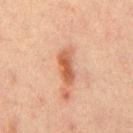Clinical impression: Part of a total-body skin-imaging series; this lesion was reviewed on a skin check and was not flagged for biopsy. Image and clinical context: A 15 mm close-up extracted from a 3D total-body photography capture. Measured at roughly 4.5 mm in maximum diameter. The total-body-photography lesion software estimated a lesion area of about 7 mm² and a shape-asymmetry score of about 0.25 (0 = symmetric). And it measured a mean CIELAB color near L≈48 a*≈22 b*≈31, about 11 CIELAB-L* units darker than the surrounding skin, and a lesion-to-skin contrast of about 8.5 (normalized; higher = more distinct). And it measured a border-irregularity index near 3/10 and peripheral color asymmetry of about 1. The analysis additionally found a nevus-likeness score of about 70/100 and lesion-presence confidence of about 100/100. The subject is a male aged 43–47. This is a cross-polarized tile. The lesion is on the chest.